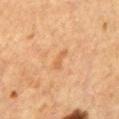Impression: The lesion was photographed on a routine skin check and not biopsied; there is no pathology result.